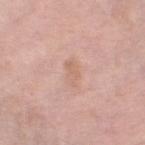No biopsy was performed on this lesion — it was imaged during a full skin examination and was not determined to be concerning.
A 15 mm close-up extracted from a 3D total-body photography capture.
A female subject aged around 65.
On the leg.